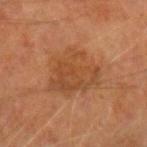  biopsy_status: not biopsied; imaged during a skin examination
  site: left forearm
  lighting: cross-polarized
  lesion_size:
    long_diameter_mm_approx: 6.5
  patient:
    sex: male
    age_approx: 65
  automated_metrics:
    area_mm2_approx: 21.0
    eccentricity: 0.5
    shape_asymmetry: 0.25
    nevus_likeness_0_100: 10
    lesion_detection_confidence_0_100: 100
  image:
    source: total-body photography crop
    field_of_view_mm: 15